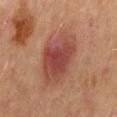{"biopsy_status": "not biopsied; imaged during a skin examination", "patient": {"sex": "male", "age_approx": 65}, "image": {"source": "total-body photography crop", "field_of_view_mm": 15}, "site": "abdomen"}